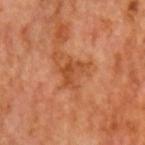Notes:
* notes — imaged on a skin check; not biopsied
* patient — male, roughly 60 years of age
* imaging modality — 15 mm crop, total-body photography
* anatomic site — the head or neck